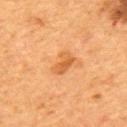<lesion>
<biopsy_status>not biopsied; imaged during a skin examination</biopsy_status>
<image>
  <source>total-body photography crop</source>
  <field_of_view_mm>15</field_of_view_mm>
</image>
<lighting>cross-polarized</lighting>
<site>back</site>
<automated_metrics>
  <area_mm2_approx>4.5</area_mm2_approx>
  <eccentricity>0.8</eccentricity>
  <shape_asymmetry>0.25</shape_asymmetry>
  <border_irregularity_0_10>2.5</border_irregularity_0_10>
</automated_metrics>
<lesion_size>
  <long_diameter_mm_approx>3.0</long_diameter_mm_approx>
</lesion_size>
<patient>
  <sex>male</sex>
  <age_approx>55</age_approx>
</patient>
</lesion>